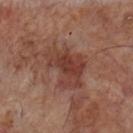notes=imaged on a skin check; not biopsied.